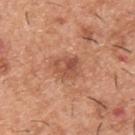Clinical impression: The lesion was photographed on a routine skin check and not biopsied; there is no pathology result. Context: The recorded lesion diameter is about 3 mm. The total-body-photography lesion software estimated a footprint of about 6.5 mm² and an eccentricity of roughly 0.55. The analysis additionally found a border-irregularity rating of about 2.5/10, a color-variation rating of about 6/10, and radial color variation of about 2.5. The analysis additionally found an automated nevus-likeness rating near 45 out of 100 and a lesion-detection confidence of about 100/100. A lesion tile, about 15 mm wide, cut from a 3D total-body photograph. On the upper back. The tile uses white-light illumination. The subject is a male aged 38–42.